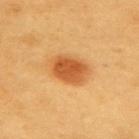biopsy status: catalogued during a skin exam; not biopsied | acquisition: ~15 mm tile from a whole-body skin photo | location: the upper back | subject: male, aged approximately 60 | lesion diameter: ~4.5 mm (longest diameter).